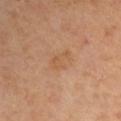Recorded during total-body skin imaging; not selected for excision or biopsy.
The patient is a male aged 63–67.
On the left upper arm.
A 15 mm close-up extracted from a 3D total-body photography capture.
This is a cross-polarized tile.
About 3 mm across.
The total-body-photography lesion software estimated a footprint of about 5 mm², an eccentricity of roughly 0.7, and a shape-asymmetry score of about 0.35 (0 = symmetric). The software also gave a lesion-detection confidence of about 100/100.